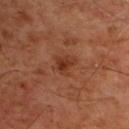Notes:
– workup — total-body-photography surveillance lesion; no biopsy
– illumination — cross-polarized
– size — about 2.5 mm
– automated lesion analysis — an eccentricity of roughly 0.45 and a symmetry-axis asymmetry near 0.45; border irregularity of about 4.5 on a 0–10 scale, a within-lesion color-variation index near 3.5/10, and a peripheral color-asymmetry measure near 1.5; a classifier nevus-likeness of about 20/100
– body site — the chest
– patient — male, aged approximately 60
– acquisition — ~15 mm tile from a whole-body skin photo